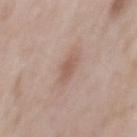Measured at roughly 3 mm in maximum diameter. A 15 mm close-up tile from a total-body photography series done for melanoma screening. A male patient, in their mid-50s. This is a white-light tile. From the mid back.